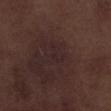The lesion was tiled from a total-body skin photograph and was not biopsied. A male patient, roughly 70 years of age. Measured at roughly 2.5 mm in maximum diameter. On the right lower leg. A 15 mm crop from a total-body photograph taken for skin-cancer surveillance.